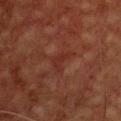Assessment:
Part of a total-body skin-imaging series; this lesion was reviewed on a skin check and was not flagged for biopsy.
Acquisition and patient details:
The total-body-photography lesion software estimated a lesion area of about 3.5 mm², an outline eccentricity of about 0.85 (0 = round, 1 = elongated), and two-axis asymmetry of about 0.55. It also reported a mean CIELAB color near L≈25 a*≈23 b*≈23 and about 4 CIELAB-L* units darker than the surrounding skin. The analysis additionally found border irregularity of about 5.5 on a 0–10 scale and a color-variation rating of about 0.5/10. It also reported a classifier nevus-likeness of about 0/100 and lesion-presence confidence of about 80/100. The lesion is located on the chest. This is a cross-polarized tile. A male patient, in their 60s. A region of skin cropped from a whole-body photographic capture, roughly 15 mm wide. The recorded lesion diameter is about 3 mm.